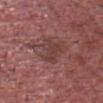Recorded during total-body skin imaging; not selected for excision or biopsy. A roughly 15 mm field-of-view crop from a total-body skin photograph. The lesion-visualizer software estimated an average lesion color of about L≈40 a*≈23 b*≈22 (CIELAB) and a lesion–skin lightness drop of about 7. It also reported a border-irregularity rating of about 6/10, a within-lesion color-variation index near 2.5/10, and peripheral color asymmetry of about 1. Measured at roughly 4 mm in maximum diameter. The patient is a male approximately 75 years of age. On the head or neck. Imaged with white-light lighting.